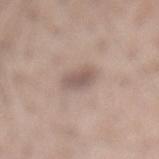tile lighting: white-light illumination | subject: male, approximately 60 years of age | imaging modality: ~15 mm tile from a whole-body skin photo | size: about 2.5 mm | anatomic site: the back | TBP lesion metrics: a mean CIELAB color near L≈55 a*≈15 b*≈21, a lesion–skin lightness drop of about 10, and a normalized lesion–skin contrast near 7; a border-irregularity index near 1.5/10 and radial color variation of about 0.5.